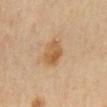Impression: Part of a total-body skin-imaging series; this lesion was reviewed on a skin check and was not flagged for biopsy. Clinical summary: The total-body-photography lesion software estimated a lesion area of about 7.5 mm². And it measured an average lesion color of about L≈48 a*≈16 b*≈33 (CIELAB) and a normalized border contrast of about 7. And it measured a border-irregularity index near 2/10 and a within-lesion color-variation index near 4/10. A close-up tile cropped from a whole-body skin photograph, about 15 mm across. The subject is a male in their mid- to late 40s. The tile uses cross-polarized illumination. On the mid back.